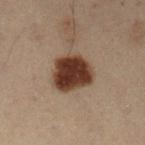No biopsy was performed on this lesion — it was imaged during a full skin examination and was not determined to be concerning. About 5 mm across. A male patient, aged around 55. From the left lower leg. A region of skin cropped from a whole-body photographic capture, roughly 15 mm wide. The tile uses cross-polarized illumination. The lesion-visualizer software estimated an outline eccentricity of about 0.6 (0 = round, 1 = elongated) and two-axis asymmetry of about 0.2. The analysis additionally found roughly 16 lightness units darker than nearby skin. It also reported a border-irregularity rating of about 2/10, a within-lesion color-variation index near 4/10, and radial color variation of about 1.5.